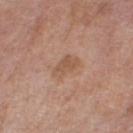Q: Was a biopsy performed?
A: imaged on a skin check; not biopsied
Q: What lighting was used for the tile?
A: white-light
Q: Patient demographics?
A: female, about 65 years old
Q: What did automated image analysis measure?
A: an area of roughly 5.5 mm², an outline eccentricity of about 0.75 (0 = round, 1 = elongated), and two-axis asymmetry of about 0.25; border irregularity of about 2.5 on a 0–10 scale, a within-lesion color-variation index near 1.5/10, and peripheral color asymmetry of about 0.5; a nevus-likeness score of about 5/100
Q: What is the imaging modality?
A: ~15 mm tile from a whole-body skin photo
Q: Lesion location?
A: the left leg
Q: How large is the lesion?
A: ~3.5 mm (longest diameter)